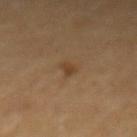follow-up: catalogued during a skin exam; not biopsied
lighting: cross-polarized illumination
site: the mid back
acquisition: ~15 mm tile from a whole-body skin photo
image-analysis metrics: an eccentricity of roughly 0.75 and two-axis asymmetry of about 0.4; border irregularity of about 3.5 on a 0–10 scale; a classifier nevus-likeness of about 40/100 and a lesion-detection confidence of about 100/100
patient: female, aged 63 to 67